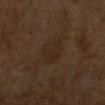Part of a total-body skin-imaging series; this lesion was reviewed on a skin check and was not flagged for biopsy.
About 4 mm across.
A male subject approximately 65 years of age.
Automated tile analysis of the lesion measured a color-variation rating of about 2/10 and a peripheral color-asymmetry measure near 0.5.
Cropped from a total-body skin-imaging series; the visible field is about 15 mm.
Imaged with cross-polarized lighting.